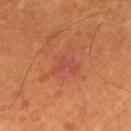follow-up: imaged on a skin check; not biopsied
automated metrics: a lesion area of about 5 mm² and a symmetry-axis asymmetry near 0.5; an automated nevus-likeness rating near 0 out of 100 and a detector confidence of about 100 out of 100 that the crop contains a lesion
site: the mid back
image: ~15 mm crop, total-body skin-cancer survey
subject: male, aged around 60